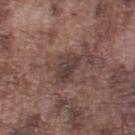Clinical impression:
The lesion was photographed on a routine skin check and not biopsied; there is no pathology result.
Clinical summary:
Approximately 3.5 mm at its widest. An algorithmic analysis of the crop reported a border-irregularity index near 2.5/10, a within-lesion color-variation index near 4/10, and a peripheral color-asymmetry measure near 1.5. It also reported a nevus-likeness score of about 5/100 and a lesion-detection confidence of about 85/100. Captured under white-light illumination. From the left lower leg. Cropped from a whole-body photographic skin survey; the tile spans about 15 mm. A male subject, aged 73 to 77.